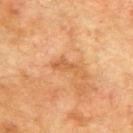  biopsy_status: not biopsied; imaged during a skin examination
  lighting: cross-polarized
  lesion_size:
    long_diameter_mm_approx: 3.5
  site: upper back
  automated_metrics:
    area_mm2_approx: 3.5
    eccentricity: 0.9
    shape_asymmetry: 0.35
    cielab_L: 49
    cielab_a: 22
    cielab_b: 36
    border_irregularity_0_10: 4.5
    color_variation_0_10: 0.5
    peripheral_color_asymmetry: 0.0
  image:
    source: total-body photography crop
    field_of_view_mm: 15
  patient:
    sex: male
    age_approx: 75